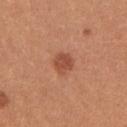Clinical impression: This lesion was catalogued during total-body skin photography and was not selected for biopsy.